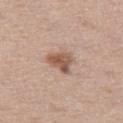<tbp_lesion>
<biopsy_status>not biopsied; imaged during a skin examination</biopsy_status>
<lighting>white-light</lighting>
<image>
  <source>total-body photography crop</source>
  <field_of_view_mm>15</field_of_view_mm>
</image>
<site>leg</site>
<automated_metrics>
  <cielab_L>55</cielab_L>
  <cielab_a>19</cielab_a>
  <cielab_b>28</cielab_b>
  <vs_skin_darker_L>12.0</vs_skin_darker_L>
  <vs_skin_contrast_norm>8.5</vs_skin_contrast_norm>
  <border_irregularity_0_10>3.5</border_irregularity_0_10>
  <color_variation_0_10>3.5</color_variation_0_10>
  <nevus_likeness_0_100>80</nevus_likeness_0_100>
  <lesion_detection_confidence_0_100>100</lesion_detection_confidence_0_100>
</automated_metrics>
<patient>
  <sex>female</sex>
  <age_approx>50</age_approx>
</patient>
</tbp_lesion>